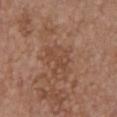Q: Was this lesion biopsied?
A: imaged on a skin check; not biopsied
Q: Patient demographics?
A: female, aged around 65
Q: Lesion size?
A: ≈4 mm
Q: Where on the body is the lesion?
A: the chest
Q: How was this image acquired?
A: 15 mm crop, total-body photography
Q: What did automated image analysis measure?
A: a shape eccentricity near 0.7; a lesion color around L≈46 a*≈20 b*≈30 in CIELAB and about 6 CIELAB-L* units darker than the surrounding skin; internal color variation of about 2.5 on a 0–10 scale and a peripheral color-asymmetry measure near 1